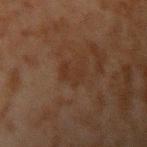Clinical impression: The lesion was photographed on a routine skin check and not biopsied; there is no pathology result. Context: A region of skin cropped from a whole-body photographic capture, roughly 15 mm wide. This is a cross-polarized tile. Approximately 3 mm at its widest. Located on the left upper arm. A male patient in their mid-40s. The total-body-photography lesion software estimated a border-irregularity index near 5.5/10, a within-lesion color-variation index near 1/10, and radial color variation of about 0.5.